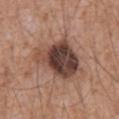Imaged during a routine full-body skin examination; the lesion was not biopsied and no histopathology is available. Measured at roughly 6 mm in maximum diameter. An algorithmic analysis of the crop reported a lesion area of about 20 mm², a shape eccentricity near 0.6, and two-axis asymmetry of about 0.35. It also reported a border-irregularity rating of about 4/10, a within-lesion color-variation index near 8/10, and a peripheral color-asymmetry measure near 2.5. Located on the mid back. The tile uses white-light illumination. A region of skin cropped from a whole-body photographic capture, roughly 15 mm wide. The patient is a male approximately 60 years of age.